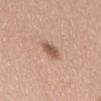Part of a total-body skin-imaging series; this lesion was reviewed on a skin check and was not flagged for biopsy.
A 15 mm crop from a total-body photograph taken for skin-cancer surveillance.
About 3 mm across.
An algorithmic analysis of the crop reported an area of roughly 4 mm² and a shape-asymmetry score of about 0.2 (0 = symmetric). The software also gave an average lesion color of about L≈55 a*≈21 b*≈29 (CIELAB), about 13 CIELAB-L* units darker than the surrounding skin, and a normalized lesion–skin contrast near 8.5.
A female subject aged 43–47.
Captured under white-light illumination.
The lesion is on the mid back.